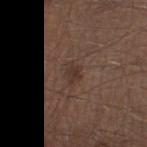biopsy_status: not biopsied; imaged during a skin examination
lesion_size:
  long_diameter_mm_approx: 2.5
patient:
  sex: male
  age_approx: 45
site: left thigh
image:
  source: total-body photography crop
  field_of_view_mm: 15
automated_metrics:
  cielab_L: 34
  cielab_a: 16
  cielab_b: 22
  vs_skin_darker_L: 6.0
  vs_skin_contrast_norm: 6.0
  nevus_likeness_0_100: 10
  lesion_detection_confidence_0_100: 100